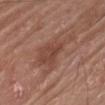Captured during whole-body skin photography for melanoma surveillance; the lesion was not biopsied. On the right forearm. A roughly 15 mm field-of-view crop from a total-body skin photograph. The subject is a male approximately 80 years of age.